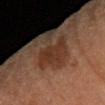{"biopsy_status": "not biopsied; imaged during a skin examination", "automated_metrics": {"vs_skin_darker_L": 6.0, "nevus_likeness_0_100": 5}, "lighting": "cross-polarized", "patient": {"sex": "female", "age_approx": 65}, "site": "left arm", "image": {"source": "total-body photography crop", "field_of_view_mm": 15}}